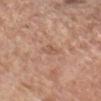| field | value |
|---|---|
| automated lesion analysis | a footprint of about 3 mm², an eccentricity of roughly 0.85, and a shape-asymmetry score of about 0.4 (0 = symmetric); roughly 7 lightness units darker than nearby skin and a lesion-to-skin contrast of about 4.5 (normalized; higher = more distinct); lesion-presence confidence of about 100/100 |
| illumination | white-light illumination |
| diameter | ≈3 mm |
| imaging modality | 15 mm crop, total-body photography |
| body site | the head or neck |
| subject | female, aged 68–72 |